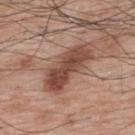<tbp_lesion>
  <biopsy_status>not biopsied; imaged during a skin examination</biopsy_status>
  <site>upper back</site>
  <patient>
    <sex>male</sex>
    <age_approx>70</age_approx>
  </patient>
  <image>
    <source>total-body photography crop</source>
    <field_of_view_mm>15</field_of_view_mm>
  </image>
</tbp_lesion>